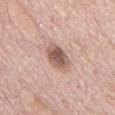follow-up: total-body-photography surveillance lesion; no biopsy | anatomic site: the mid back | patient: male, aged approximately 75 | image source: ~15 mm crop, total-body skin-cancer survey.